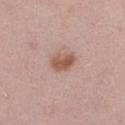Captured during whole-body skin photography for melanoma surveillance; the lesion was not biopsied.
The recorded lesion diameter is about 3 mm.
A 15 mm crop from a total-body photograph taken for skin-cancer surveillance.
The patient is a male in their 40s.
Located on the right thigh.